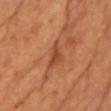| key | value |
|---|---|
| diameter | about 4 mm |
| image-analysis metrics | a classifier nevus-likeness of about 0/100 and lesion-presence confidence of about 60/100 |
| location | the front of the torso |
| imaging modality | ~15 mm tile from a whole-body skin photo |
| lighting | cross-polarized illumination |
| patient | female, aged 68 to 72 |